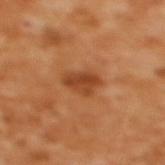  biopsy_status: not biopsied; imaged during a skin examination
  site: upper back
  lesion_size:
    long_diameter_mm_approx: 3.5
  image:
    source: total-body photography crop
    field_of_view_mm: 15
  patient:
    sex: female
    age_approx: 55
  lighting: cross-polarized